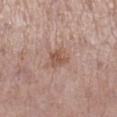This image is a 15 mm lesion crop taken from a total-body photograph. A female patient aged around 50. On the right lower leg. The lesion-visualizer software estimated a classifier nevus-likeness of about 10/100 and a detector confidence of about 100 out of 100 that the crop contains a lesion. This is a white-light tile.